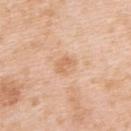Clinical impression:
Captured during whole-body skin photography for melanoma surveillance; the lesion was not biopsied.
Image and clinical context:
Cropped from a total-body skin-imaging series; the visible field is about 15 mm. The patient is a female in their 40s. The tile uses white-light illumination. The lesion is on the upper back.